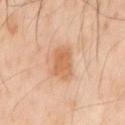No biopsy was performed on this lesion — it was imaged during a full skin examination and was not determined to be concerning. This is a cross-polarized tile. A male subject, aged 53–57. Cropped from a whole-body photographic skin survey; the tile spans about 15 mm. Automated tile analysis of the lesion measured an area of roughly 8.5 mm², an outline eccentricity of about 0.8 (0 = round, 1 = elongated), and a shape-asymmetry score of about 0.2 (0 = symmetric). And it measured a mean CIELAB color near L≈63 a*≈23 b*≈37 and a normalized border contrast of about 7. The software also gave border irregularity of about 2.5 on a 0–10 scale and a within-lesion color-variation index near 2.5/10. The software also gave a classifier nevus-likeness of about 40/100 and a detector confidence of about 100 out of 100 that the crop contains a lesion. The lesion's longest dimension is about 4 mm. Located on the mid back.